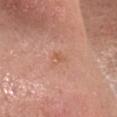The lesion was tiled from a total-body skin photograph and was not biopsied.
The lesion is on the head or neck.
The patient is a female about 45 years old.
A lesion tile, about 15 mm wide, cut from a 3D total-body photograph.